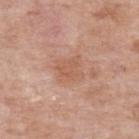This lesion was catalogued during total-body skin photography and was not selected for biopsy. Located on the left forearm. Captured under white-light illumination. The subject is a female aged around 70. Cropped from a whole-body photographic skin survey; the tile spans about 15 mm. Measured at roughly 3 mm in maximum diameter.